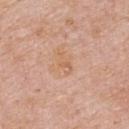{
  "biopsy_status": "not biopsied; imaged during a skin examination",
  "site": "right upper arm",
  "patient": {
    "sex": "male",
    "age_approx": 75
  },
  "image": {
    "source": "total-body photography crop",
    "field_of_view_mm": 15
  }
}